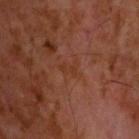Case summary:
* lighting: cross-polarized illumination
* image source: total-body-photography crop, ~15 mm field of view
* lesion diameter: ~2.5 mm (longest diameter)
* anatomic site: the head or neck
* patient: male, aged 58 to 62
* automated metrics: an eccentricity of roughly 0.8 and a symmetry-axis asymmetry near 0.5